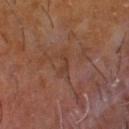<tbp_lesion>
  <biopsy_status>not biopsied; imaged during a skin examination</biopsy_status>
  <patient>
    <sex>male</sex>
    <age_approx>60</age_approx>
  </patient>
  <image>
    <source>total-body photography crop</source>
    <field_of_view_mm>15</field_of_view_mm>
  </image>
  <site>left lower leg</site>
</tbp_lesion>